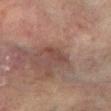Clinical impression: No biopsy was performed on this lesion — it was imaged during a full skin examination and was not determined to be concerning. Image and clinical context: The lesion is located on the left arm. Automated tile analysis of the lesion measured a lesion color around L≈40 a*≈19 b*≈22 in CIELAB and roughly 7 lightness units darker than nearby skin. A 15 mm close-up extracted from a 3D total-body photography capture. A female patient, in their 80s.